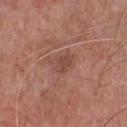Imaged during a routine full-body skin examination; the lesion was not biopsied and no histopathology is available.
Located on the chest.
Imaged with white-light lighting.
A 15 mm close-up extracted from a 3D total-body photography capture.
A male patient aged 68 to 72.